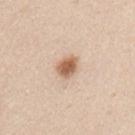A lesion tile, about 15 mm wide, cut from a 3D total-body photograph.
A male patient about 45 years old.
Measured at roughly 3 mm in maximum diameter.
Captured under white-light illumination.
The lesion is on the chest.